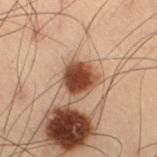The lesion was tiled from a total-body skin photograph and was not biopsied.
Imaged with cross-polarized lighting.
A male subject, aged 53–57.
The lesion is on the right thigh.
Measured at roughly 3 mm in maximum diameter.
A 15 mm close-up extracted from a 3D total-body photography capture.
Automated image analysis of the tile measured a lesion area of about 8.5 mm², an eccentricity of roughly 0.25, and a symmetry-axis asymmetry near 0.15. The software also gave a nevus-likeness score of about 100/100.